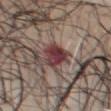No biopsy was performed on this lesion — it was imaged during a full skin examination and was not determined to be concerning. Measured at roughly 4.5 mm in maximum diameter. The lesion-visualizer software estimated a footprint of about 7.5 mm², a shape eccentricity near 0.85, and a shape-asymmetry score of about 0.3 (0 = symmetric). It also reported a mean CIELAB color near L≈38 a*≈23 b*≈17 and a normalized border contrast of about 13. The patient is a male about 70 years old. Captured under white-light illumination. Cropped from a whole-body photographic skin survey; the tile spans about 15 mm. The lesion is on the front of the torso.